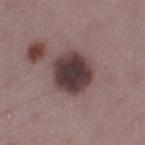Case summary:
• subject: female, aged approximately 45
• site: the left thigh
• acquisition: total-body-photography crop, ~15 mm field of view